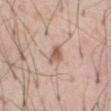The patient is a male about 55 years old. Automated image analysis of the tile measured a border-irregularity rating of about 4/10. A roughly 15 mm field-of-view crop from a total-body skin photograph. From the abdomen. Imaged with white-light lighting.